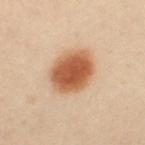The lesion was tiled from a total-body skin photograph and was not biopsied. This image is a 15 mm lesion crop taken from a total-body photograph. The patient is a female approximately 40 years of age. Approximately 5.5 mm at its widest. Captured under cross-polarized illumination. The lesion is located on the left upper arm. An algorithmic analysis of the crop reported an area of roughly 18 mm² and two-axis asymmetry of about 0.1. It also reported a classifier nevus-likeness of about 100/100 and a lesion-detection confidence of about 100/100.